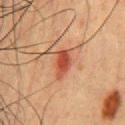Assessment:
The lesion was photographed on a routine skin check and not biopsied; there is no pathology result.
Background:
A male subject aged 48 to 52. Imaged with cross-polarized lighting. Located on the front of the torso. A lesion tile, about 15 mm wide, cut from a 3D total-body photograph.